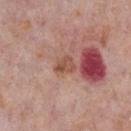<record>
<biopsy_status>not biopsied; imaged during a skin examination</biopsy_status>
<automated_metrics>
  <cielab_L>52</cielab_L>
  <cielab_a>22</cielab_a>
  <cielab_b>28</cielab_b>
  <vs_skin_darker_L>9.0</vs_skin_darker_L>
  <vs_skin_contrast_norm>7.0</vs_skin_contrast_norm>
  <lesion_detection_confidence_0_100>100</lesion_detection_confidence_0_100>
</automated_metrics>
<site>chest</site>
<lesion_size>
  <long_diameter_mm_approx>2.5</long_diameter_mm_approx>
</lesion_size>
<image>
  <source>total-body photography crop</source>
  <field_of_view_mm>15</field_of_view_mm>
</image>
<patient>
  <sex>male</sex>
  <age_approx>75</age_approx>
</patient>
</record>